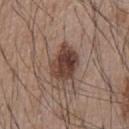Impression:
No biopsy was performed on this lesion — it was imaged during a full skin examination and was not determined to be concerning.
Acquisition and patient details:
Imaged with white-light lighting. The patient is a male about 55 years old. A 15 mm close-up tile from a total-body photography series done for melanoma screening. Approximately 5 mm at its widest. The lesion is on the chest.